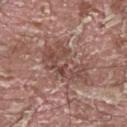Q: Was a biopsy performed?
A: total-body-photography surveillance lesion; no biopsy
Q: What is the anatomic site?
A: the upper back
Q: Patient demographics?
A: male, aged around 40
Q: How was this image acquired?
A: total-body-photography crop, ~15 mm field of view
Q: Lesion size?
A: ≈6 mm